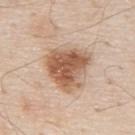Recorded during total-body skin imaging; not selected for excision or biopsy.
The lesion-visualizer software estimated a border-irregularity rating of about 3.5/10 and radial color variation of about 2. The analysis additionally found a classifier nevus-likeness of about 95/100.
Measured at roughly 5 mm in maximum diameter.
Captured under white-light illumination.
Cropped from a total-body skin-imaging series; the visible field is about 15 mm.
A male subject aged 78 to 82.
The lesion is located on the upper back.